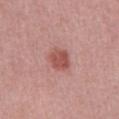Impression:
Imaged during a routine full-body skin examination; the lesion was not biopsied and no histopathology is available.
Context:
A male patient aged approximately 50. This image is a 15 mm lesion crop taken from a total-body photograph. The lesion is located on the front of the torso.